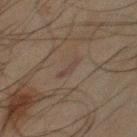Q: Was this lesion biopsied?
A: total-body-photography surveillance lesion; no biopsy
Q: Where on the body is the lesion?
A: the chest
Q: How was this image acquired?
A: ~15 mm crop, total-body skin-cancer survey
Q: Who is the patient?
A: male, in their mid- to late 40s
Q: Lesion size?
A: ~3 mm (longest diameter)